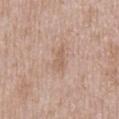| feature | finding |
|---|---|
| biopsy status | no biopsy performed (imaged during a skin exam) |
| site | the chest |
| diameter | about 3 mm |
| acquisition | ~15 mm tile from a whole-body skin photo |
| patient | male, aged around 50 |
| tile lighting | white-light illumination |
| TBP lesion metrics | an eccentricity of roughly 0.9 and a symmetry-axis asymmetry near 0.45; a lesion color around L≈60 a*≈18 b*≈29 in CIELAB, a lesion–skin lightness drop of about 7, and a normalized border contrast of about 5; a border-irregularity index near 4.5/10, a within-lesion color-variation index near 1/10, and radial color variation of about 0.5; a detector confidence of about 100 out of 100 that the crop contains a lesion |